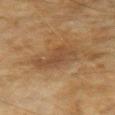{"biopsy_status": "not biopsied; imaged during a skin examination", "patient": {"sex": "female", "age_approx": 60}, "lighting": "cross-polarized", "site": "arm", "image": {"source": "total-body photography crop", "field_of_view_mm": 15}, "lesion_size": {"long_diameter_mm_approx": 4.5}}